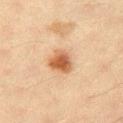Part of a total-body skin-imaging series; this lesion was reviewed on a skin check and was not flagged for biopsy. The subject is a female about 40 years old. Cropped from a whole-body photographic skin survey; the tile spans about 15 mm. The lesion is on the left thigh.